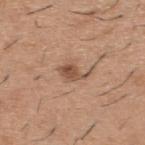Q: Automated lesion metrics?
A: an area of roughly 5 mm², an eccentricity of roughly 0.8, and a shape-asymmetry score of about 0.4 (0 = symmetric); a mean CIELAB color near L≈52 a*≈19 b*≈30 and a lesion–skin lightness drop of about 11
Q: How was this image acquired?
A: total-body-photography crop, ~15 mm field of view
Q: What is the lesion's diameter?
A: ~3.5 mm (longest diameter)
Q: What is the anatomic site?
A: the upper back
Q: Who is the patient?
A: male, about 40 years old
Q: What lighting was used for the tile?
A: white-light